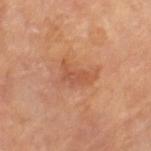Approximately 4 mm at its widest. The lesion is on the left thigh. A female subject aged 68 to 72. Cropped from a total-body skin-imaging series; the visible field is about 15 mm. Captured under cross-polarized illumination.